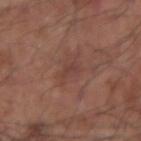notes = catalogued during a skin exam; not biopsied
lesion diameter = about 2.5 mm
illumination = white-light
image source = ~15 mm crop, total-body skin-cancer survey
patient = male, aged 53–57
body site = the arm